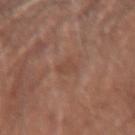| field | value |
|---|---|
| notes | imaged on a skin check; not biopsied |
| image source | ~15 mm crop, total-body skin-cancer survey |
| patient | male, aged around 65 |
| anatomic site | the right forearm |